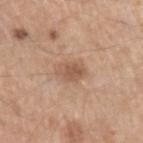Q: Was this lesion biopsied?
A: no biopsy performed (imaged during a skin exam)
Q: Lesion location?
A: the arm
Q: What is the imaging modality?
A: 15 mm crop, total-body photography
Q: How large is the lesion?
A: ≈3 mm
Q: What are the patient's age and sex?
A: male, roughly 55 years of age